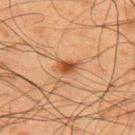biopsy status = total-body-photography surveillance lesion; no biopsy | diameter = about 2.5 mm | site = the upper back | imaging modality = ~15 mm crop, total-body skin-cancer survey | image-analysis metrics = a color-variation rating of about 4.5/10 and peripheral color asymmetry of about 1.5 | subject = male, approximately 60 years of age.